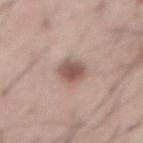- notes: no biopsy performed (imaged during a skin exam)
- body site: the abdomen
- lighting: white-light illumination
- patient: male, about 55 years old
- image: ~15 mm crop, total-body skin-cancer survey
- lesion size: about 3.5 mm